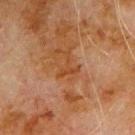biopsy status: no biopsy performed (imaged during a skin exam)
image source: ~15 mm crop, total-body skin-cancer survey
subject: male, about 80 years old
anatomic site: the left upper arm
automated lesion analysis: a footprint of about 3.5 mm², an outline eccentricity of about 0.7 (0 = round, 1 = elongated), and a symmetry-axis asymmetry near 0.75; a normalized lesion–skin contrast near 6; internal color variation of about 0 on a 0–10 scale and peripheral color asymmetry of about 0